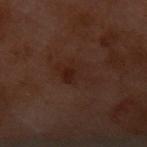The lesion was tiled from a total-body skin photograph and was not biopsied.
Located on the front of the torso.
An algorithmic analysis of the crop reported border irregularity of about 6.5 on a 0–10 scale, internal color variation of about 3 on a 0–10 scale, and peripheral color asymmetry of about 1.
Measured at roughly 5 mm in maximum diameter.
The subject is a female in their 60s.
This image is a 15 mm lesion crop taken from a total-body photograph.
Captured under cross-polarized illumination.